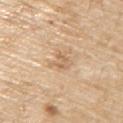workup — total-body-photography surveillance lesion; no biopsy | patient — male, approximately 70 years of age | lesion diameter — about 2.5 mm | imaging modality — 15 mm crop, total-body photography | image-analysis metrics — an eccentricity of roughly 0.4 and two-axis asymmetry of about 0.4; a border-irregularity index near 5/10, a within-lesion color-variation index near 3.5/10, and peripheral color asymmetry of about 1 | location — the upper back.